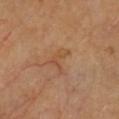This lesion was catalogued during total-body skin photography and was not selected for biopsy.
The patient is a female aged 68 to 72.
From the chest.
The tile uses cross-polarized illumination.
An algorithmic analysis of the crop reported a footprint of about 4.5 mm². It also reported a lesion color around L≈51 a*≈22 b*≈35 in CIELAB, about 6 CIELAB-L* units darker than the surrounding skin, and a normalized border contrast of about 5. And it measured border irregularity of about 7.5 on a 0–10 scale and peripheral color asymmetry of about 0.
A lesion tile, about 15 mm wide, cut from a 3D total-body photograph.